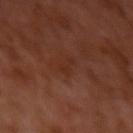Impression:
Part of a total-body skin-imaging series; this lesion was reviewed on a skin check and was not flagged for biopsy.
Acquisition and patient details:
The tile uses cross-polarized illumination. The lesion-visualizer software estimated an area of roughly 2.5 mm², an eccentricity of roughly 0.95, and a symmetry-axis asymmetry near 0.55. The software also gave an automated nevus-likeness rating near 0 out of 100 and lesion-presence confidence of about 95/100. A male patient aged approximately 30. Longest diameter approximately 3 mm. Cropped from a whole-body photographic skin survey; the tile spans about 15 mm. The lesion is on the left upper arm.